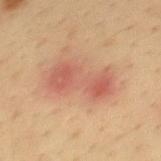biopsy status — no biopsy performed (imaged during a skin exam); subject — male, aged 33 to 37; tile lighting — cross-polarized; imaging modality — 15 mm crop, total-body photography; lesion diameter — ≈7 mm; anatomic site — the back.